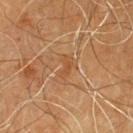Findings:
- image-analysis metrics: a lesion area of about 3 mm², a shape eccentricity near 0.9, and a symmetry-axis asymmetry near 0.45; roughly 6 lightness units darker than nearby skin; a border-irregularity rating of about 4.5/10 and peripheral color asymmetry of about 0
- illumination: cross-polarized
- acquisition: ~15 mm crop, total-body skin-cancer survey
- lesion diameter: ~3 mm (longest diameter)
- subject: male, approximately 60 years of age
- body site: the chest